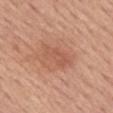Findings:
– biopsy status: catalogued during a skin exam; not biopsied
– anatomic site: the back
– image: ~15 mm crop, total-body skin-cancer survey
– lesion diameter: about 4.5 mm
– subject: female, about 65 years old
– tile lighting: white-light illumination